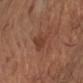The lesion was tiled from a total-body skin photograph and was not biopsied.
A region of skin cropped from a whole-body photographic capture, roughly 15 mm wide.
The lesion is on the arm.
The subject is a male roughly 55 years of age.